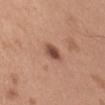Part of a total-body skin-imaging series; this lesion was reviewed on a skin check and was not flagged for biopsy. The recorded lesion diameter is about 2.5 mm. Located on the right upper arm. A 15 mm close-up tile from a total-body photography series done for melanoma screening. An algorithmic analysis of the crop reported a border-irregularity rating of about 2/10, a color-variation rating of about 3.5/10, and a peripheral color-asymmetry measure near 1. This is a white-light tile. A male subject aged 53 to 57.